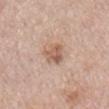Findings:
– notes — catalogued during a skin exam; not biopsied
– size — about 3 mm
– anatomic site — the abdomen
– tile lighting — white-light illumination
– automated metrics — a shape eccentricity near 0.6 and a shape-asymmetry score of about 0.3 (0 = symmetric); an average lesion color of about L≈59 a*≈19 b*≈29 (CIELAB), about 11 CIELAB-L* units darker than the surrounding skin, and a normalized border contrast of about 7; internal color variation of about 3 on a 0–10 scale; a classifier nevus-likeness of about 10/100
– image — 15 mm crop, total-body photography
– patient — male, aged approximately 75